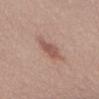Part of a total-body skin-imaging series; this lesion was reviewed on a skin check and was not flagged for biopsy.
Automated tile analysis of the lesion measured a lesion color around L≈53 a*≈22 b*≈25 in CIELAB, a lesion–skin lightness drop of about 10, and a normalized border contrast of about 6.5. The analysis additionally found border irregularity of about 2.5 on a 0–10 scale and a within-lesion color-variation index near 1.5/10. The analysis additionally found a detector confidence of about 100 out of 100 that the crop contains a lesion.
The lesion is located on the lower back.
Cropped from a whole-body photographic skin survey; the tile spans about 15 mm.
The subject is a female aged 53 to 57.